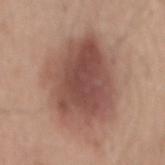Clinical impression:
Imaged during a routine full-body skin examination; the lesion was not biopsied and no histopathology is available.
Clinical summary:
The lesion is on the mid back. A male patient about 45 years old. A close-up tile cropped from a whole-body skin photograph, about 15 mm across.